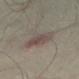follow-up: no biopsy performed (imaged during a skin exam) | automated lesion analysis: an area of roughly 9.5 mm², a shape eccentricity near 0.8, and two-axis asymmetry of about 0.15 | body site: the abdomen | image: total-body-photography crop, ~15 mm field of view | lesion diameter: ~4 mm (longest diameter) | subject: male, in their mid- to late 50s.